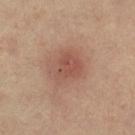Part of a total-body skin-imaging series; this lesion was reviewed on a skin check and was not flagged for biopsy. This is a cross-polarized tile. A female patient roughly 40 years of age. Located on the right thigh. Cropped from a total-body skin-imaging series; the visible field is about 15 mm.